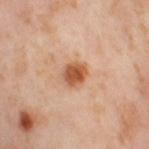follow-up — imaged on a skin check; not biopsied
tile lighting — cross-polarized
automated lesion analysis — a lesion area of about 5.5 mm², an outline eccentricity of about 0.6 (0 = round, 1 = elongated), and a shape-asymmetry score of about 0.2 (0 = symmetric); a lesion color around L≈55 a*≈25 b*≈37 in CIELAB and roughly 14 lightness units darker than nearby skin; a classifier nevus-likeness of about 95/100 and a lesion-detection confidence of about 100/100
image — ~15 mm crop, total-body skin-cancer survey
location — the right thigh
diameter — ~3 mm (longest diameter)
patient — female, aged around 55